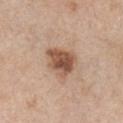notes: total-body-photography surveillance lesion; no biopsy | diameter: ≈4 mm | site: the front of the torso | subject: male, in their mid- to late 70s | lighting: white-light illumination | imaging modality: ~15 mm tile from a whole-body skin photo.